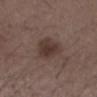<record>
  <automated_metrics>
    <border_irregularity_0_10>2.5</border_irregularity_0_10>
    <peripheral_color_asymmetry>0.5</peripheral_color_asymmetry>
  </automated_metrics>
  <lesion_size>
    <long_diameter_mm_approx>3.5</long_diameter_mm_approx>
  </lesion_size>
  <image>
    <source>total-body photography crop</source>
    <field_of_view_mm>15</field_of_view_mm>
  </image>
  <patient>
    <sex>male</sex>
    <age_approx>50</age_approx>
  </patient>
  <lighting>white-light</lighting>
  <site>lower back</site>
</record>